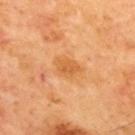| field | value |
|---|---|
| notes | no biopsy performed (imaged during a skin exam) |
| diameter | ~3.5 mm (longest diameter) |
| patient | male, aged 63 to 67 |
| site | the upper back |
| imaging modality | 15 mm crop, total-body photography |
| illumination | cross-polarized |